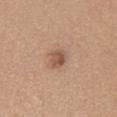| feature | finding |
|---|---|
| follow-up | total-body-photography surveillance lesion; no biopsy |
| body site | the chest |
| image-analysis metrics | a footprint of about 4 mm², an eccentricity of roughly 0.75, and a symmetry-axis asymmetry near 0.15; a border-irregularity index near 1.5/10, a color-variation rating of about 2.5/10, and peripheral color asymmetry of about 1; an automated nevus-likeness rating near 80 out of 100 and a lesion-detection confidence of about 100/100 |
| illumination | white-light |
| acquisition | total-body-photography crop, ~15 mm field of view |
| subject | male, aged 58–62 |
| lesion diameter | about 2.5 mm |